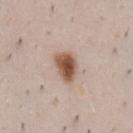Recorded during total-body skin imaging; not selected for excision or biopsy.
The lesion's longest dimension is about 4 mm.
A close-up tile cropped from a whole-body skin photograph, about 15 mm across.
The lesion is on the front of the torso.
This is a white-light tile.
A male patient about 50 years old.